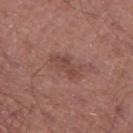The lesion was photographed on a routine skin check and not biopsied; there is no pathology result.